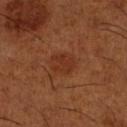follow-up — no biopsy performed (imaged during a skin exam)
patient — male, in their mid-60s
site — the leg
acquisition — ~15 mm tile from a whole-body skin photo